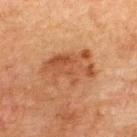  biopsy_status: not biopsied; imaged during a skin examination
  lesion_size:
    long_diameter_mm_approx: 6.0
  site: back
  image:
    source: total-body photography crop
    field_of_view_mm: 15
  patient:
    sex: male
    age_approx: 75